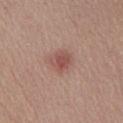{"image": {"source": "total-body photography crop", "field_of_view_mm": 15}, "patient": {"sex": "male", "age_approx": 55}, "site": "abdomen", "lesion_size": {"long_diameter_mm_approx": 2.5}, "automated_metrics": {"area_mm2_approx": 5.5, "eccentricity": 0.4, "cielab_L": 51, "cielab_a": 23, "cielab_b": 24, "vs_skin_darker_L": 9.0, "vs_skin_contrast_norm": 6.5, "border_irregularity_0_10": 2.0, "color_variation_0_10": 2.5, "nevus_likeness_0_100": 60}}